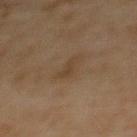Q: What is the imaging modality?
A: 15 mm crop, total-body photography
Q: Lesion location?
A: the back
Q: Who is the patient?
A: female, aged approximately 70